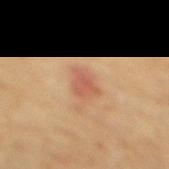* notes · no biopsy performed (imaged during a skin exam)
* site · the mid back
* image · ~15 mm tile from a whole-body skin photo
* lesion diameter · ≈3 mm
* subject · male, roughly 70 years of age
* illumination · cross-polarized